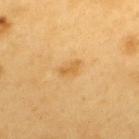The lesion was tiled from a total-body skin photograph and was not biopsied. This is a cross-polarized tile. On the upper back. A lesion tile, about 15 mm wide, cut from a 3D total-body photograph. A male subject roughly 60 years of age.